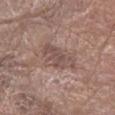Part of a total-body skin-imaging series; this lesion was reviewed on a skin check and was not flagged for biopsy. On the left forearm. A female patient, aged around 80. The lesion's longest dimension is about 4.5 mm. The tile uses white-light illumination. A lesion tile, about 15 mm wide, cut from a 3D total-body photograph. Automated image analysis of the tile measured an eccentricity of roughly 0.8.